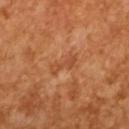{"biopsy_status": "not biopsied; imaged during a skin examination", "image": {"source": "total-body photography crop", "field_of_view_mm": 15}, "patient": {"sex": "male", "age_approx": 65}, "lesion_size": {"long_diameter_mm_approx": 3.5}, "lighting": "cross-polarized"}